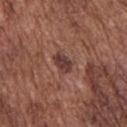Part of a total-body skin-imaging series; this lesion was reviewed on a skin check and was not flagged for biopsy.
The tile uses white-light illumination.
A male patient approximately 75 years of age.
A roughly 15 mm field-of-view crop from a total-body skin photograph.
The lesion is located on the upper back.